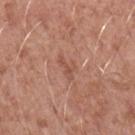- notes: no biopsy performed (imaged during a skin exam)
- lighting: white-light
- automated metrics: a border-irregularity rating of about 5/10, a color-variation rating of about 2.5/10, and radial color variation of about 1; a classifier nevus-likeness of about 0/100 and a lesion-detection confidence of about 100/100
- patient: male, about 50 years old
- image source: 15 mm crop, total-body photography
- site: the arm